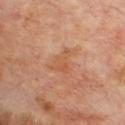A 15 mm close-up tile from a total-body photography series done for melanoma screening. Located on the chest. The patient is a male in their 70s.